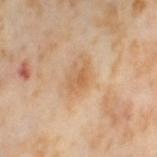The lesion's longest dimension is about 3.5 mm.
An algorithmic analysis of the crop reported a lesion area of about 4 mm², an outline eccentricity of about 0.8 (0 = round, 1 = elongated), and a symmetry-axis asymmetry near 0.35. The software also gave roughly 8 lightness units darker than nearby skin and a normalized lesion–skin contrast near 6. And it measured border irregularity of about 4 on a 0–10 scale and internal color variation of about 2 on a 0–10 scale. The analysis additionally found an automated nevus-likeness rating near 0 out of 100 and a detector confidence of about 100 out of 100 that the crop contains a lesion.
A close-up tile cropped from a whole-body skin photograph, about 15 mm across.
Imaged with cross-polarized lighting.
A female subject aged 53–57.
From the left thigh.